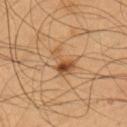image:
  source: total-body photography crop
  field_of_view_mm: 15
patient:
  sex: male
  age_approx: 55
lesion_size:
  long_diameter_mm_approx: 4.0
site: arm